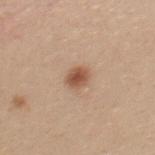Clinical impression: Captured during whole-body skin photography for melanoma surveillance; the lesion was not biopsied. Context: An algorithmic analysis of the crop reported an outline eccentricity of about 0.5 (0 = round, 1 = elongated) and a shape-asymmetry score of about 0.15 (0 = symmetric). It also reported a within-lesion color-variation index near 2.5/10 and a peripheral color-asymmetry measure near 1. The software also gave an automated nevus-likeness rating near 100 out of 100 and lesion-presence confidence of about 100/100. From the upper back. Imaged with white-light lighting. The subject is a female aged approximately 25. A 15 mm close-up tile from a total-body photography series done for melanoma screening.